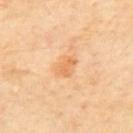Imaged during a routine full-body skin examination; the lesion was not biopsied and no histopathology is available. A lesion tile, about 15 mm wide, cut from a 3D total-body photograph. The patient is a male aged 63–67. About 2.5 mm across. The lesion is located on the mid back. The lesion-visualizer software estimated a lesion area of about 4 mm² and a shape-asymmetry score of about 0.3 (0 = symmetric). It also reported a border-irregularity index near 2.5/10 and radial color variation of about 0.5. It also reported an automated nevus-likeness rating near 5 out of 100 and lesion-presence confidence of about 100/100. The tile uses cross-polarized illumination.